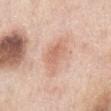Recorded during total-body skin imaging; not selected for excision or biopsy.
The tile uses white-light illumination.
A 15 mm crop from a total-body photograph taken for skin-cancer surveillance.
On the abdomen.
About 5.5 mm across.
A male subject roughly 75 years of age.